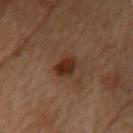follow-up=total-body-photography surveillance lesion; no biopsy
imaging modality=~15 mm tile from a whole-body skin photo
size=~3 mm (longest diameter)
lighting=cross-polarized
automated lesion analysis=a lesion area of about 5.5 mm² and a symmetry-axis asymmetry near 0.15; a lesion color around L≈26 a*≈18 b*≈25 in CIELAB; a border-irregularity index near 1.5/10 and a peripheral color-asymmetry measure near 1
subject=female, approximately 60 years of age
anatomic site=the head or neck